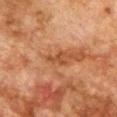Impression: This lesion was catalogued during total-body skin photography and was not selected for biopsy. Acquisition and patient details: The subject is a male in their mid- to late 70s. The lesion is located on the chest. This image is a 15 mm lesion crop taken from a total-body photograph.